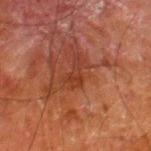biopsy_status: not biopsied; imaged during a skin examination
automated_metrics:
  vs_skin_darker_L: 5.0
  vs_skin_contrast_norm: 5.5
  border_irregularity_0_10: 6.0
  color_variation_0_10: 1.0
  peripheral_color_asymmetry: 0.0
  nevus_likeness_0_100: 0
  lesion_detection_confidence_0_100: 95
patient:
  sex: male
  age_approx: 80
lighting: cross-polarized
lesion_size:
  long_diameter_mm_approx: 3.0
image:
  source: total-body photography crop
  field_of_view_mm: 15
site: leg